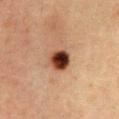This lesion was catalogued during total-body skin photography and was not selected for biopsy. A 15 mm close-up tile from a total-body photography series done for melanoma screening. A female patient, aged approximately 40. Captured under cross-polarized illumination. Measured at roughly 2.5 mm in maximum diameter. Located on the chest.